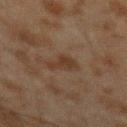automated lesion analysis — roughly 6 lightness units darker than nearby skin and a normalized border contrast of about 6.5; a detector confidence of about 100 out of 100 that the crop contains a lesion | image source — ~15 mm tile from a whole-body skin photo | anatomic site — the left forearm | subject — male, in their mid-40s | lesion diameter — ~3.5 mm (longest diameter) | lighting — cross-polarized illumination.